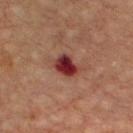Q: Was this lesion biopsied?
A: no biopsy performed (imaged during a skin exam)
Q: What is the imaging modality?
A: ~15 mm tile from a whole-body skin photo
Q: Where on the body is the lesion?
A: the upper back
Q: Patient demographics?
A: male, about 65 years old
Q: What lighting was used for the tile?
A: cross-polarized
Q: Automated lesion metrics?
A: a shape eccentricity near 0.6 and a symmetry-axis asymmetry near 0.25; an average lesion color of about L≈31 a*≈28 b*≈22 (CIELAB) and about 15 CIELAB-L* units darker than the surrounding skin; a border-irregularity index near 2/10, internal color variation of about 5.5 on a 0–10 scale, and peripheral color asymmetry of about 1.5; a classifier nevus-likeness of about 0/100 and lesion-presence confidence of about 100/100
Q: Lesion size?
A: about 3 mm